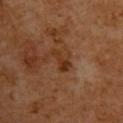Impression: Captured during whole-body skin photography for melanoma surveillance; the lesion was not biopsied. Clinical summary: The subject is a male aged around 60. A 15 mm close-up tile from a total-body photography series done for melanoma screening. The lesion is on the upper back.